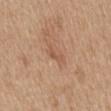This lesion was catalogued during total-body skin photography and was not selected for biopsy.
A female patient aged around 55.
On the mid back.
A roughly 15 mm field-of-view crop from a total-body skin photograph.